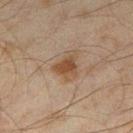| field | value |
|---|---|
| biopsy status | no biopsy performed (imaged during a skin exam) |
| location | the right thigh |
| image source | 15 mm crop, total-body photography |
| automated metrics | an area of roughly 5 mm², an eccentricity of roughly 0.55, and two-axis asymmetry of about 0.35; a border-irregularity rating of about 3/10 and a color-variation rating of about 1.5/10; a classifier nevus-likeness of about 70/100 and a lesion-detection confidence of about 100/100 |
| patient | male, approximately 45 years of age |
| lighting | cross-polarized |
| size | ≈3 mm |